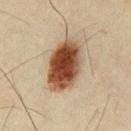Clinical impression: Part of a total-body skin-imaging series; this lesion was reviewed on a skin check and was not flagged for biopsy. Acquisition and patient details: An algorithmic analysis of the crop reported an outline eccentricity of about 0.8 (0 = round, 1 = elongated) and a symmetry-axis asymmetry near 0.1. It also reported a mean CIELAB color near L≈43 a*≈19 b*≈30 and a lesion–skin lightness drop of about 19. A male patient, in their 50s. The lesion is located on the chest. The lesion's longest dimension is about 6.5 mm. A region of skin cropped from a whole-body photographic capture, roughly 15 mm wide. The tile uses cross-polarized illumination.